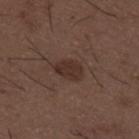Clinical impression:
Captured during whole-body skin photography for melanoma surveillance; the lesion was not biopsied.
Acquisition and patient details:
On the mid back. A male subject aged 48 to 52. Imaged with white-light lighting. A 15 mm crop from a total-body photograph taken for skin-cancer surveillance. The total-body-photography lesion software estimated an average lesion color of about L≈30 a*≈16 b*≈22 (CIELAB), a lesion–skin lightness drop of about 7, and a normalized lesion–skin contrast near 7.5. It also reported a border-irregularity rating of about 2/10, a color-variation rating of about 2/10, and a peripheral color-asymmetry measure near 1.